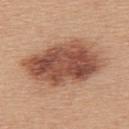Recorded during total-body skin imaging; not selected for excision or biopsy. A region of skin cropped from a whole-body photographic capture, roughly 15 mm wide. Located on the upper back. Measured at roughly 10 mm in maximum diameter. The patient is a female aged 43 to 47. This is a white-light tile.